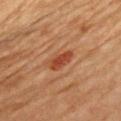<lesion>
  <biopsy_status>not biopsied; imaged during a skin examination</biopsy_status>
  <patient>
    <sex>male</sex>
    <age_approx>70</age_approx>
  </patient>
  <site>chest</site>
  <image>
    <source>total-body photography crop</source>
    <field_of_view_mm>15</field_of_view_mm>
  </image>
</lesion>